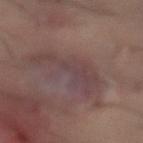This lesion was catalogued during total-body skin photography and was not selected for biopsy. Located on the abdomen. Imaged with cross-polarized lighting. A 15 mm close-up tile from a total-body photography series done for melanoma screening. The lesion's longest dimension is about 7 mm. The subject is a male in their 60s.